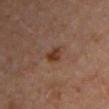Assessment: Captured during whole-body skin photography for melanoma surveillance; the lesion was not biopsied. Clinical summary: Measured at roughly 2.5 mm in maximum diameter. The tile uses cross-polarized illumination. The lesion is on the right upper arm. An algorithmic analysis of the crop reported an area of roughly 4 mm² and two-axis asymmetry of about 0.3. And it measured an average lesion color of about L≈35 a*≈20 b*≈28 (CIELAB), a lesion–skin lightness drop of about 9, and a normalized lesion–skin contrast near 9. The software also gave a lesion-detection confidence of about 100/100. A region of skin cropped from a whole-body photographic capture, roughly 15 mm wide. The patient is a female roughly 50 years of age.